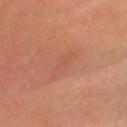No biopsy was performed on this lesion — it was imaged during a full skin examination and was not determined to be concerning.
From the head or neck.
Imaged with cross-polarized lighting.
The patient is a male in their mid-40s.
A region of skin cropped from a whole-body photographic capture, roughly 15 mm wide.